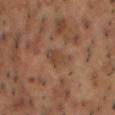| key | value |
|---|---|
| notes | catalogued during a skin exam; not biopsied |
| tile lighting | cross-polarized |
| automated metrics | a border-irregularity index near 3.5/10, internal color variation of about 3 on a 0–10 scale, and radial color variation of about 1; a nevus-likeness score of about 0/100 and a lesion-detection confidence of about 80/100 |
| acquisition | ~15 mm tile from a whole-body skin photo |
| site | the head or neck |
| lesion size | about 3.5 mm |
| patient | male, aged approximately 55 |